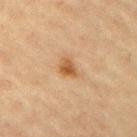lesion_size:
  long_diameter_mm_approx: 3.0
lighting: cross-polarized
automated_metrics:
  nevus_likeness_0_100: 90
image:
  source: total-body photography crop
  field_of_view_mm: 15
site: arm
patient:
  sex: female
  age_approx: 65